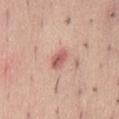| feature | finding |
|---|---|
| workup | catalogued during a skin exam; not biopsied |
| illumination | white-light illumination |
| body site | the lower back |
| image source | 15 mm crop, total-body photography |
| automated lesion analysis | a nevus-likeness score of about 5/100 and a detector confidence of about 100 out of 100 that the crop contains a lesion |
| diameter | ~2.5 mm (longest diameter) |
| patient | female, aged 33 to 37 |